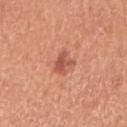workup: total-body-photography surveillance lesion; no biopsy | image source: ~15 mm crop, total-body skin-cancer survey | lesion diameter: ≈2.5 mm | tile lighting: white-light illumination | location: the right upper arm | patient: female, about 60 years old.